The lesion is on the mid back, cropped from a whole-body photographic skin survey; the tile spans about 15 mm, the subject is a male aged 38 to 42 — 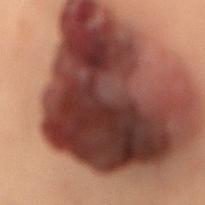About 13.5 mm across.
This is a cross-polarized tile.
Histopathologically confirmed as an invasive melanoma, Breslow thickness 1.8 mm and mitotic rate >4/mm² — a malignant skin lesion.